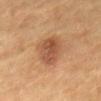{"biopsy_status": "not biopsied; imaged during a skin examination", "lighting": "cross-polarized", "site": "back", "image": {"source": "total-body photography crop", "field_of_view_mm": 15}, "patient": {"sex": "male", "age_approx": "85+"}, "lesion_size": {"long_diameter_mm_approx": 4.0}}